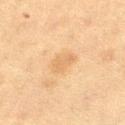Impression:
Captured during whole-body skin photography for melanoma surveillance; the lesion was not biopsied.
Context:
A male patient, aged approximately 55. Located on the right thigh. A roughly 15 mm field-of-view crop from a total-body skin photograph. The tile uses cross-polarized illumination.